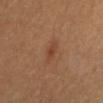<lesion>
<biopsy_status>not biopsied; imaged during a skin examination</biopsy_status>
<lesion_size>
  <long_diameter_mm_approx>3.0</long_diameter_mm_approx>
</lesion_size>
<site>abdomen</site>
<image>
  <source>total-body photography crop</source>
  <field_of_view_mm>15</field_of_view_mm>
</image>
<patient>
  <sex>male</sex>
  <age_approx>60</age_approx>
</patient>
</lesion>